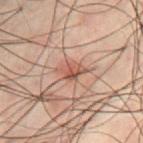Findings:
– follow-up — catalogued during a skin exam; not biopsied
– patient — male, aged 48 to 52
– size — about 3 mm
– lighting — cross-polarized illumination
– acquisition — ~15 mm crop, total-body skin-cancer survey
– location — the abdomen